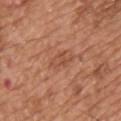This lesion was catalogued during total-body skin photography and was not selected for biopsy.
The total-body-photography lesion software estimated about 7 CIELAB-L* units darker than the surrounding skin and a normalized lesion–skin contrast near 5. It also reported border irregularity of about 4 on a 0–10 scale, a within-lesion color-variation index near 2.5/10, and peripheral color asymmetry of about 1.
Imaged with white-light lighting.
This image is a 15 mm lesion crop taken from a total-body photograph.
The subject is a male aged around 65.
Located on the chest.
The recorded lesion diameter is about 2.5 mm.